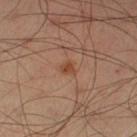Notes:
* notes: total-body-photography surveillance lesion; no biopsy
* TBP lesion metrics: a lesion color around L≈45 a*≈23 b*≈32 in CIELAB and a normalized lesion–skin contrast near 7.5; a classifier nevus-likeness of about 85/100 and lesion-presence confidence of about 100/100
* subject: male, in their mid-50s
* image: ~15 mm tile from a whole-body skin photo
* body site: the left lower leg
* diameter: ~2 mm (longest diameter)